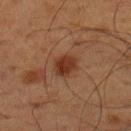Case summary:
– follow-up — imaged on a skin check; not biopsied
– body site — the upper back
– TBP lesion metrics — an area of roughly 6 mm² and a symmetry-axis asymmetry near 0.2; a lesion color around L≈26 a*≈19 b*≈25 in CIELAB, roughly 8 lightness units darker than nearby skin, and a normalized lesion–skin contrast near 9; border irregularity of about 2 on a 0–10 scale; a nevus-likeness score of about 85/100
– image source — total-body-photography crop, ~15 mm field of view
– patient — male, in their 60s